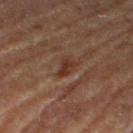workup = catalogued during a skin exam; not biopsied | image source = total-body-photography crop, ~15 mm field of view | body site = the leg | patient = male, in their mid- to late 80s or older.